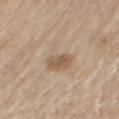Recorded during total-body skin imaging; not selected for excision or biopsy.
A 15 mm close-up extracted from a 3D total-body photography capture.
From the right thigh.
The total-body-photography lesion software estimated an average lesion color of about L≈60 a*≈13 b*≈30 (CIELAB) and a normalized lesion–skin contrast near 5. It also reported a nevus-likeness score of about 55/100 and a detector confidence of about 100 out of 100 that the crop contains a lesion.
Imaged with white-light lighting.
A male subject about 70 years old.
Approximately 5 mm at its widest.